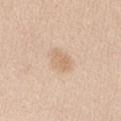The lesion was tiled from a total-body skin photograph and was not biopsied.